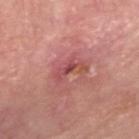Recorded during total-body skin imaging; not selected for excision or biopsy.
Longest diameter approximately 3 mm.
The lesion is located on the head or neck.
Cropped from a total-body skin-imaging series; the visible field is about 15 mm.
A male patient, in their mid- to late 60s.